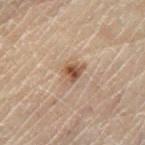Recorded during total-body skin imaging; not selected for excision or biopsy. The lesion's longest dimension is about 2.5 mm. Imaged with cross-polarized lighting. A male subject aged approximately 70. A roughly 15 mm field-of-view crop from a total-body skin photograph. On the left lower leg.